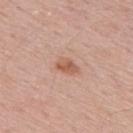Part of a total-body skin-imaging series; this lesion was reviewed on a skin check and was not flagged for biopsy. Automated tile analysis of the lesion measured an average lesion color of about L≈58 a*≈22 b*≈31 (CIELAB), about 10 CIELAB-L* units darker than the surrounding skin, and a lesion-to-skin contrast of about 7 (normalized; higher = more distinct). A close-up tile cropped from a whole-body skin photograph, about 15 mm across. Located on the upper back. The patient is a male in their mid-50s.